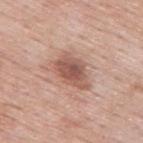Imaged during a routine full-body skin examination; the lesion was not biopsied and no histopathology is available.
A male subject, in their mid- to late 50s.
A lesion tile, about 15 mm wide, cut from a 3D total-body photograph.
Located on the back.